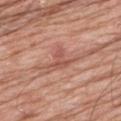acquisition — ~15 mm crop, total-body skin-cancer survey
patient — male, about 80 years old
location — the back
diameter — ~3 mm (longest diameter)
illumination — white-light